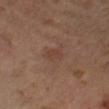Captured during whole-body skin photography for melanoma surveillance; the lesion was not biopsied. A male patient about 70 years old. A close-up tile cropped from a whole-body skin photograph, about 15 mm across. This is a cross-polarized tile. An algorithmic analysis of the crop reported a lesion color around L≈40 a*≈18 b*≈26 in CIELAB, about 5 CIELAB-L* units darker than the surrounding skin, and a normalized border contrast of about 4.5. The analysis additionally found an automated nevus-likeness rating near 0 out of 100. On the right lower leg.